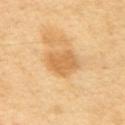The lesion was photographed on a routine skin check and not biopsied; there is no pathology result. Captured under cross-polarized illumination. Longest diameter approximately 4 mm. A female subject approximately 50 years of age. A 15 mm crop from a total-body photograph taken for skin-cancer surveillance. Located on the left upper arm.